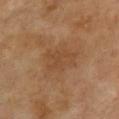Impression:
No biopsy was performed on this lesion — it was imaged during a full skin examination and was not determined to be concerning.
Image and clinical context:
Automated tile analysis of the lesion measured an average lesion color of about L≈41 a*≈18 b*≈31 (CIELAB). Imaged with cross-polarized lighting. A 15 mm close-up extracted from a 3D total-body photography capture. The patient is a female about 60 years old. The lesion is on the chest. Measured at roughly 4.5 mm in maximum diameter.